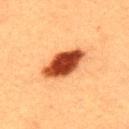Q: Was a biopsy performed?
A: total-body-photography surveillance lesion; no biopsy
Q: Who is the patient?
A: male, aged 38 to 42
Q: How was this image acquired?
A: ~15 mm tile from a whole-body skin photo
Q: Where on the body is the lesion?
A: the upper back
Q: How was the tile lit?
A: cross-polarized illumination
Q: Automated lesion metrics?
A: a mean CIELAB color near L≈41 a*≈29 b*≈36, a lesion–skin lightness drop of about 22, and a normalized border contrast of about 15.5
Q: How large is the lesion?
A: ~5.5 mm (longest diameter)